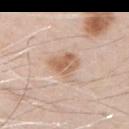Impression: Recorded during total-body skin imaging; not selected for excision or biopsy. Image and clinical context: Automated tile analysis of the lesion measured a footprint of about 8 mm², an eccentricity of roughly 0.55, and two-axis asymmetry of about 0.3. It also reported roughly 10 lightness units darker than nearby skin and a lesion-to-skin contrast of about 7.5 (normalized; higher = more distinct). It also reported a border-irregularity index near 3/10, a within-lesion color-variation index near 4/10, and radial color variation of about 1.5. From the left upper arm. A male patient, in their 70s. A lesion tile, about 15 mm wide, cut from a 3D total-body photograph.